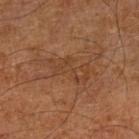workup: total-body-photography surveillance lesion; no biopsy
subject: male, roughly 60 years of age
tile lighting: cross-polarized
image source: ~15 mm crop, total-body skin-cancer survey
body site: the right leg
lesion size: about 5.5 mm
automated metrics: a lesion area of about 9.5 mm², a shape eccentricity near 0.9, and two-axis asymmetry of about 0.4; a border-irregularity rating of about 6/10, a within-lesion color-variation index near 2.5/10, and peripheral color asymmetry of about 1